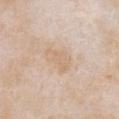Clinical impression: Recorded during total-body skin imaging; not selected for excision or biopsy.